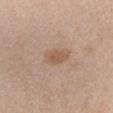Part of a total-body skin-imaging series; this lesion was reviewed on a skin check and was not flagged for biopsy. About 3 mm across. Captured under white-light illumination. The total-body-photography lesion software estimated an area of roughly 5 mm² and a shape eccentricity near 0.8. The analysis additionally found a mean CIELAB color near L≈56 a*≈17 b*≈30 and roughly 8 lightness units darker than nearby skin. The analysis additionally found a nevus-likeness score of about 10/100 and a detector confidence of about 100 out of 100 that the crop contains a lesion. The lesion is located on the chest. A 15 mm close-up tile from a total-body photography series done for melanoma screening. A female subject in their mid-20s.